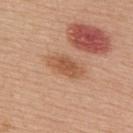No biopsy was performed on this lesion — it was imaged during a full skin examination and was not determined to be concerning. This image is a 15 mm lesion crop taken from a total-body photograph. A male subject aged around 55. Located on the upper back.